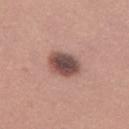Q: Was this lesion biopsied?
A: total-body-photography surveillance lesion; no biopsy
Q: What is the imaging modality?
A: total-body-photography crop, ~15 mm field of view
Q: Who is the patient?
A: female, in their 30s
Q: How large is the lesion?
A: ~4 mm (longest diameter)
Q: What lighting was used for the tile?
A: white-light
Q: Lesion location?
A: the left thigh
Q: Automated lesion metrics?
A: a footprint of about 9.5 mm², a shape eccentricity near 0.75, and a shape-asymmetry score of about 0.1 (0 = symmetric)